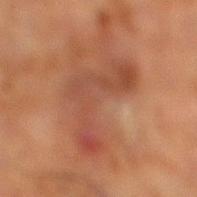Imaged during a routine full-body skin examination; the lesion was not biopsied and no histopathology is available. Located on the right lower leg. A male patient roughly 70 years of age. Cropped from a total-body skin-imaging series; the visible field is about 15 mm.